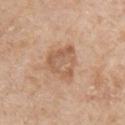This lesion was catalogued during total-body skin photography and was not selected for biopsy. Cropped from a total-body skin-imaging series; the visible field is about 15 mm. A male patient aged 63 to 67. The lesion is located on the chest.